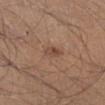workup: total-body-photography surveillance lesion; no biopsy
acquisition: 15 mm crop, total-body photography
patient: male, approximately 45 years of age
lesion diameter: about 2.5 mm
anatomic site: the left lower leg